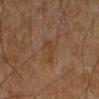biopsy status: no biopsy performed (imaged during a skin exam) | subject: male, roughly 45 years of age | TBP lesion metrics: an average lesion color of about L≈31 a*≈15 b*≈25 (CIELAB) and roughly 5 lightness units darker than nearby skin; an automated nevus-likeness rating near 0 out of 100 and lesion-presence confidence of about 100/100 | size: ≈2.5 mm | site: the left forearm | illumination: cross-polarized illumination | imaging modality: ~15 mm crop, total-body skin-cancer survey.